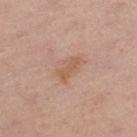Q: Is there a histopathology result?
A: no biopsy performed (imaged during a skin exam)
Q: Lesion location?
A: the right lower leg
Q: Who is the patient?
A: female, about 65 years old
Q: What kind of image is this?
A: total-body-photography crop, ~15 mm field of view